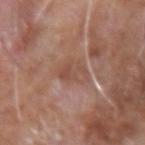follow-up: imaged on a skin check; not biopsied
lesion size: about 4 mm
patient: male, aged around 75
lighting: white-light illumination
site: the arm
image: 15 mm crop, total-body photography